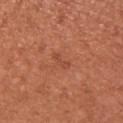Captured during whole-body skin photography for melanoma surveillance; the lesion was not biopsied.
The lesion-visualizer software estimated a lesion–skin lightness drop of about 6. The analysis additionally found a border-irregularity rating of about 5/10, a color-variation rating of about 0/10, and a peripheral color-asymmetry measure near 0. And it measured lesion-presence confidence of about 100/100.
From the upper back.
A roughly 15 mm field-of-view crop from a total-body skin photograph.
Imaged with white-light lighting.
A female subject, in their 30s.